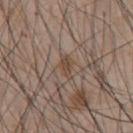Impression: The lesion was tiled from a total-body skin photograph and was not biopsied. Background: Located on the chest. A male subject, aged 43–47. Measured at roughly 2.5 mm in maximum diameter. This image is a 15 mm lesion crop taken from a total-body photograph. The total-body-photography lesion software estimated a lesion area of about 3.5 mm², a shape eccentricity near 0.85, and a symmetry-axis asymmetry near 0.4. And it measured an automated nevus-likeness rating near 0 out of 100 and a detector confidence of about 90 out of 100 that the crop contains a lesion. This is a white-light tile.